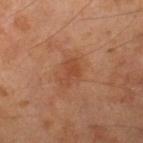Q: Was a biopsy performed?
A: no biopsy performed (imaged during a skin exam)